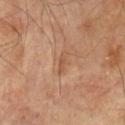Q: What lighting was used for the tile?
A: cross-polarized illumination
Q: What are the patient's age and sex?
A: male, aged 73 to 77
Q: Automated lesion metrics?
A: a footprint of about 2 mm² and a symmetry-axis asymmetry near 0.4; a mean CIELAB color near L≈44 a*≈18 b*≈29, a lesion–skin lightness drop of about 6, and a normalized lesion–skin contrast near 5.5; a border-irregularity index near 5/10, internal color variation of about 0 on a 0–10 scale, and a peripheral color-asymmetry measure near 0; lesion-presence confidence of about 100/100
Q: How was this image acquired?
A: total-body-photography crop, ~15 mm field of view
Q: Lesion location?
A: the arm
Q: How large is the lesion?
A: ≈3 mm